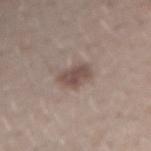{
  "biopsy_status": "not biopsied; imaged during a skin examination",
  "site": "right upper arm",
  "image": {
    "source": "total-body photography crop",
    "field_of_view_mm": 15
  },
  "patient": {
    "sex": "male",
    "age_approx": 30
  },
  "lesion_size": {
    "long_diameter_mm_approx": 3.0
  }
}